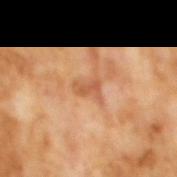Part of a total-body skin-imaging series; this lesion was reviewed on a skin check and was not flagged for biopsy. The tile uses cross-polarized illumination. The lesion-visualizer software estimated a border-irregularity rating of about 4/10, a color-variation rating of about 1.5/10, and radial color variation of about 0.5. It also reported an automated nevus-likeness rating near 0 out of 100 and a detector confidence of about 100 out of 100 that the crop contains a lesion. A 15 mm crop from a total-body photograph taken for skin-cancer surveillance. The recorded lesion diameter is about 2.5 mm. The subject is a male in their mid- to late 60s.